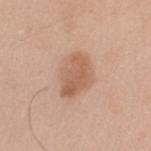follow-up: no biopsy performed (imaged during a skin exam)
lighting: white-light
lesion size: about 4.5 mm
patient: male, in their 70s
image source: ~15 mm crop, total-body skin-cancer survey
image-analysis metrics: an eccentricity of roughly 0.75 and a symmetry-axis asymmetry near 0.2; roughly 10 lightness units darker than nearby skin; border irregularity of about 2 on a 0–10 scale, internal color variation of about 3.5 on a 0–10 scale, and a peripheral color-asymmetry measure near 1; an automated nevus-likeness rating near 70 out of 100 and lesion-presence confidence of about 100/100
location: the left upper arm